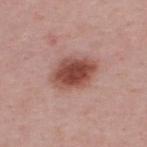Part of a total-body skin-imaging series; this lesion was reviewed on a skin check and was not flagged for biopsy.
The lesion's longest dimension is about 5 mm.
A region of skin cropped from a whole-body photographic capture, roughly 15 mm wide.
This is a white-light tile.
A male patient in their 50s.
On the upper back.